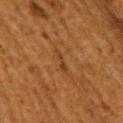  biopsy_status: not biopsied; imaged during a skin examination
  lighting: cross-polarized
  lesion_size:
    long_diameter_mm_approx: 3.0
  site: right upper arm
  patient:
    sex: female
    age_approx: 50
  image:
    source: total-body photography crop
    field_of_view_mm: 15